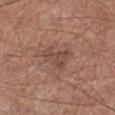<record>
<biopsy_status>not biopsied; imaged during a skin examination</biopsy_status>
<automated_metrics>
  <shape_asymmetry>0.5</shape_asymmetry>
  <nevus_likeness_0_100>0</nevus_likeness_0_100>
  <lesion_detection_confidence_0_100>100</lesion_detection_confidence_0_100>
</automated_metrics>
<lighting>white-light</lighting>
<image>
  <source>total-body photography crop</source>
  <field_of_view_mm>15</field_of_view_mm>
</image>
<site>leg</site>
<patient>
  <sex>male</sex>
  <age_approx>65</age_approx>
</patient>
</record>